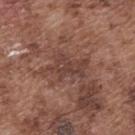Q: Is there a histopathology result?
A: no biopsy performed (imaged during a skin exam)
Q: How was the tile lit?
A: white-light illumination
Q: What is the imaging modality?
A: ~15 mm tile from a whole-body skin photo
Q: What is the lesion's diameter?
A: about 5.5 mm
Q: Who is the patient?
A: male, aged 73–77
Q: What did automated image analysis measure?
A: a border-irregularity rating of about 7.5/10 and internal color variation of about 2.5 on a 0–10 scale; a classifier nevus-likeness of about 0/100 and a lesion-detection confidence of about 65/100
Q: Lesion location?
A: the upper back